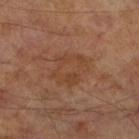• biopsy status · imaged on a skin check; not biopsied
• image source · ~15 mm crop, total-body skin-cancer survey
• tile lighting · cross-polarized illumination
• location · the right lower leg
• patient · male, about 70 years old
• size · ≈4.5 mm